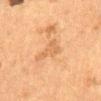{
  "biopsy_status": "not biopsied; imaged during a skin examination",
  "site": "front of the torso",
  "patient": {
    "sex": "female",
    "age_approx": 50
  },
  "image": {
    "source": "total-body photography crop",
    "field_of_view_mm": 15
  },
  "lesion_size": {
    "long_diameter_mm_approx": 3.0
  },
  "automated_metrics": {
    "eccentricity": 0.7,
    "shape_asymmetry": 0.6,
    "cielab_L": 53,
    "cielab_a": 20,
    "cielab_b": 35,
    "vs_skin_darker_L": 6.0,
    "vs_skin_contrast_norm": 4.5,
    "border_irregularity_0_10": 6.0,
    "color_variation_0_10": 1.5,
    "peripheral_color_asymmetry": 0.5
  },
  "lighting": "cross-polarized"
}